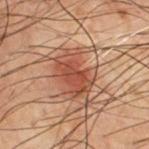biopsy status = imaged on a skin check; not biopsied
automated lesion analysis = a lesion area of about 15 mm², an eccentricity of roughly 0.7, and a symmetry-axis asymmetry near 0.25; border irregularity of about 3 on a 0–10 scale, a within-lesion color-variation index near 3.5/10, and a peripheral color-asymmetry measure near 1; an automated nevus-likeness rating near 85 out of 100 and a lesion-detection confidence of about 100/100
lesion size = about 5 mm
illumination = cross-polarized
image source = ~15 mm tile from a whole-body skin photo
patient = male, aged around 70
anatomic site = the chest